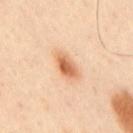Q: Was this lesion biopsied?
A: total-body-photography surveillance lesion; no biopsy
Q: Lesion location?
A: the mid back
Q: What is the lesion's diameter?
A: about 4 mm
Q: How was the tile lit?
A: cross-polarized
Q: Patient demographics?
A: male, aged 43 to 47
Q: What is the imaging modality?
A: total-body-photography crop, ~15 mm field of view